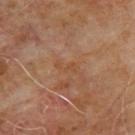- subject · male, aged 63 to 67
- anatomic site · the upper back
- acquisition · ~15 mm tile from a whole-body skin photo
- lesion diameter · about 2.5 mm